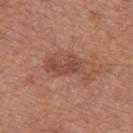This lesion was catalogued during total-body skin photography and was not selected for biopsy.
This image is a 15 mm lesion crop taken from a total-body photograph.
The tile uses white-light illumination.
On the left thigh.
Approximately 5.5 mm at its widest.
The subject is a female approximately 40 years of age.
The total-body-photography lesion software estimated a mean CIELAB color near L≈48 a*≈24 b*≈29, a lesion–skin lightness drop of about 8, and a lesion-to-skin contrast of about 6 (normalized; higher = more distinct).